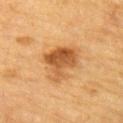Notes:
• follow-up · catalogued during a skin exam; not biopsied
• subject · male, approximately 85 years of age or older
• acquisition · 15 mm crop, total-body photography
• location · the left upper arm